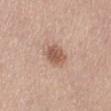The lesion was photographed on a routine skin check and not biopsied; there is no pathology result.
The lesion is on the lower back.
Automated image analysis of the tile measured an outline eccentricity of about 0.45 (0 = round, 1 = elongated) and a symmetry-axis asymmetry near 0.25. The analysis additionally found an automated nevus-likeness rating near 90 out of 100 and lesion-presence confidence of about 100/100.
A lesion tile, about 15 mm wide, cut from a 3D total-body photograph.
This is a white-light tile.
The lesion's longest dimension is about 3 mm.
A male patient about 75 years old.